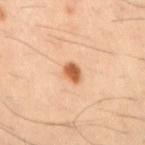* biopsy status · no biopsy performed (imaged during a skin exam)
* lesion size · ~2.5 mm (longest diameter)
* image source · ~15 mm tile from a whole-body skin photo
* lighting · cross-polarized
* site · the right upper arm
* subject · male, aged 38–42